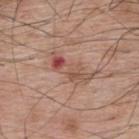No biopsy was performed on this lesion — it was imaged during a full skin examination and was not determined to be concerning.
Located on the upper back.
A region of skin cropped from a whole-body photographic capture, roughly 15 mm wide.
The subject is a male approximately 70 years of age.